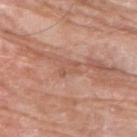Q: Was this lesion biopsied?
A: imaged on a skin check; not biopsied
Q: Illumination type?
A: white-light illumination
Q: Who is the patient?
A: male, aged approximately 65
Q: What is the imaging modality?
A: total-body-photography crop, ~15 mm field of view
Q: Lesion location?
A: the upper back
Q: Automated lesion metrics?
A: a lesion area of about 3.5 mm², an outline eccentricity of about 0.8 (0 = round, 1 = elongated), and a symmetry-axis asymmetry near 0.4; a mean CIELAB color near L≈55 a*≈23 b*≈30 and a normalized lesion–skin contrast near 5